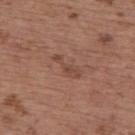– notes · catalogued during a skin exam; not biopsied